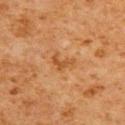follow-up: total-body-photography surveillance lesion; no biopsy | size: about 2.5 mm | location: the upper back | subject: male, roughly 60 years of age | image: ~15 mm tile from a whole-body skin photo | lighting: cross-polarized | image-analysis metrics: a border-irregularity index near 6.5/10, internal color variation of about 0 on a 0–10 scale, and radial color variation of about 0.